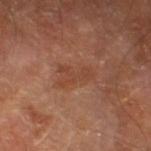The lesion was tiled from a total-body skin photograph and was not biopsied. Automated tile analysis of the lesion measured a footprint of about 5 mm² and a symmetry-axis asymmetry near 0.65. The analysis additionally found a mean CIELAB color near L≈42 a*≈24 b*≈30, about 5 CIELAB-L* units darker than the surrounding skin, and a normalized border contrast of about 5. It also reported a border-irregularity rating of about 10/10, a within-lesion color-variation index near 0.5/10, and peripheral color asymmetry of about 0. Measured at roughly 4 mm in maximum diameter. From the left thigh. A 15 mm close-up extracted from a 3D total-body photography capture. The tile uses cross-polarized illumination. A male subject, about 70 years old.